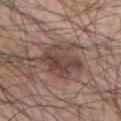Q: Is there a histopathology result?
A: no biopsy performed (imaged during a skin exam)
Q: Where on the body is the lesion?
A: the front of the torso
Q: What kind of image is this?
A: 15 mm crop, total-body photography
Q: Who is the patient?
A: male, approximately 70 years of age
Q: How large is the lesion?
A: about 6 mm
Q: What did automated image analysis measure?
A: a lesion area of about 16 mm² and a shape eccentricity near 0.7; a lesion-to-skin contrast of about 8 (normalized; higher = more distinct); an automated nevus-likeness rating near 15 out of 100 and a lesion-detection confidence of about 95/100